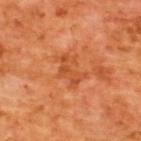  biopsy_status: not biopsied; imaged during a skin examination
  patient:
    sex: male
    age_approx: 65
  automated_metrics:
    cielab_L: 51
    cielab_a: 32
    cielab_b: 43
    vs_skin_darker_L: 7.0
    vs_skin_contrast_norm: 5.5
    border_irregularity_0_10: 7.5
    peripheral_color_asymmetry: 1.0
  site: upper back
  lighting: cross-polarized
  image:
    source: total-body photography crop
    field_of_view_mm: 15